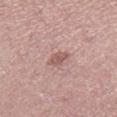Recorded during total-body skin imaging; not selected for excision or biopsy. Measured at roughly 2.5 mm in maximum diameter. Imaged with white-light lighting. On the right lower leg. The lesion-visualizer software estimated a mean CIELAB color near L≈56 a*≈21 b*≈23, about 10 CIELAB-L* units darker than the surrounding skin, and a normalized border contrast of about 6.5. The software also gave a border-irregularity index near 2.5/10 and a color-variation rating of about 1/10. A female subject, roughly 40 years of age. A 15 mm close-up tile from a total-body photography series done for melanoma screening.